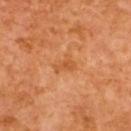Imaged during a routine full-body skin examination; the lesion was not biopsied and no histopathology is available. The tile uses cross-polarized illumination. From the back. The recorded lesion diameter is about 2.5 mm. This image is a 15 mm lesion crop taken from a total-body photograph. A female patient, aged approximately 65.